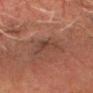• workup — total-body-photography surveillance lesion; no biopsy
• patient — male, aged 68 to 72
• body site — the head or neck
• image — ~15 mm crop, total-body skin-cancer survey
• automated metrics — a border-irregularity index near 4/10 and a color-variation rating of about 3.5/10; an automated nevus-likeness rating near 0 out of 100 and a lesion-detection confidence of about 50/100
• lighting — cross-polarized
• lesion size — ~3.5 mm (longest diameter)